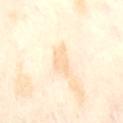Impression:
Part of a total-body skin-imaging series; this lesion was reviewed on a skin check and was not flagged for biopsy.
Context:
Approximately 4 mm at its widest. A female patient, aged approximately 55. A region of skin cropped from a whole-body photographic capture, roughly 15 mm wide. The lesion is located on the front of the torso. The tile uses cross-polarized illumination. The total-body-photography lesion software estimated an outline eccentricity of about 0.9 (0 = round, 1 = elongated).